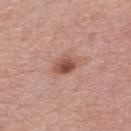biopsy status = catalogued during a skin exam; not biopsied
body site = the upper back
lesion size = about 3.5 mm
image source = ~15 mm crop, total-body skin-cancer survey
automated lesion analysis = an eccentricity of roughly 0.7; a lesion color around L≈52 a*≈24 b*≈28 in CIELAB, about 12 CIELAB-L* units darker than the surrounding skin, and a normalized border contrast of about 9; a nevus-likeness score of about 90/100 and a lesion-detection confidence of about 100/100
lighting = white-light illumination
subject = female, aged 48 to 52